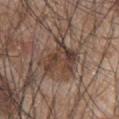No biopsy was performed on this lesion — it was imaged during a full skin examination and was not determined to be concerning. The total-body-photography lesion software estimated a peripheral color-asymmetry measure near 2. The software also gave lesion-presence confidence of about 60/100. Approximately 6 mm at its widest. Located on the upper back. The patient is a male in their mid-40s. A lesion tile, about 15 mm wide, cut from a 3D total-body photograph. This is a white-light tile.